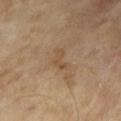No biopsy was performed on this lesion — it was imaged during a full skin examination and was not determined to be concerning.
A 15 mm crop from a total-body photograph taken for skin-cancer surveillance.
A male subject, approximately 65 years of age.